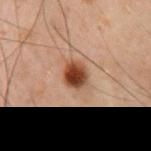Assessment:
The lesion was photographed on a routine skin check and not biopsied; there is no pathology result.
Context:
The lesion is located on the chest. A lesion tile, about 15 mm wide, cut from a 3D total-body photograph. A male patient aged 68–72. About 3 mm across. The tile uses cross-polarized illumination.